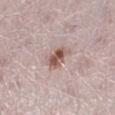Findings:
* follow-up — total-body-photography surveillance lesion; no biopsy
* patient — female, aged 48–52
* diameter — ~3.5 mm (longest diameter)
* site — the right lower leg
* imaging modality — 15 mm crop, total-body photography
* tile lighting — white-light illumination